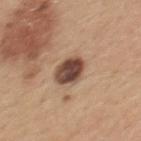Assessment:
The lesion was photographed on a routine skin check and not biopsied; there is no pathology result.
Acquisition and patient details:
The lesion's longest dimension is about 3.5 mm. The tile uses white-light illumination. The lesion is on the upper back. The subject is a female aged approximately 45. A close-up tile cropped from a whole-body skin photograph, about 15 mm across.